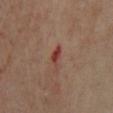notes=catalogued during a skin exam; not biopsied | body site=the chest | subject=female, aged 48–52 | image=total-body-photography crop, ~15 mm field of view | illumination=cross-polarized illumination | TBP lesion metrics=border irregularity of about 2.5 on a 0–10 scale; an automated nevus-likeness rating near 0 out of 100 and lesion-presence confidence of about 100/100.